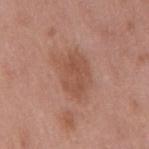biopsy status = imaged on a skin check; not biopsied | body site = the mid back | automated metrics = a border-irregularity index near 3/10 and a peripheral color-asymmetry measure near 1 | imaging modality = ~15 mm tile from a whole-body skin photo | subject = male, about 50 years old.